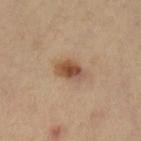Q: Is there a histopathology result?
A: no biopsy performed (imaged during a skin exam)
Q: How was the tile lit?
A: cross-polarized illumination
Q: Where on the body is the lesion?
A: the left lower leg
Q: Patient demographics?
A: female, aged 58–62
Q: What is the imaging modality?
A: ~15 mm crop, total-body skin-cancer survey